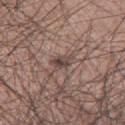Q: Was a biopsy performed?
A: catalogued during a skin exam; not biopsied